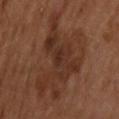Notes:
• automated lesion analysis — a lesion area of about 39 mm² and a shape-asymmetry score of about 0.5 (0 = symmetric); a lesion color around L≈30 a*≈19 b*≈26 in CIELAB, about 7 CIELAB-L* units darker than the surrounding skin, and a normalized lesion–skin contrast near 7
• imaging modality — ~15 mm crop, total-body skin-cancer survey
• subject — male, in their mid-60s
• location — the head or neck